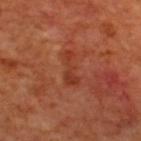Assessment: No biopsy was performed on this lesion — it was imaged during a full skin examination and was not determined to be concerning. Image and clinical context: Measured at roughly 4 mm in maximum diameter. On the back. A lesion tile, about 15 mm wide, cut from a 3D total-body photograph. The subject is a male about 70 years old. This is a cross-polarized tile. The total-body-photography lesion software estimated a mean CIELAB color near L≈37 a*≈29 b*≈33, roughly 6 lightness units darker than nearby skin, and a normalized lesion–skin contrast near 6. The software also gave a border-irregularity index near 5/10, internal color variation of about 2 on a 0–10 scale, and radial color variation of about 0.5. It also reported a classifier nevus-likeness of about 0/100.